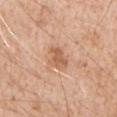notes=total-body-photography surveillance lesion; no biopsy | automated lesion analysis=a footprint of about 5.5 mm², an eccentricity of roughly 0.7, and two-axis asymmetry of about 0.25 | patient=male, roughly 60 years of age | image=15 mm crop, total-body photography | tile lighting=white-light | body site=the left upper arm.